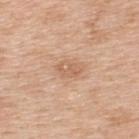This lesion was catalogued during total-body skin photography and was not selected for biopsy. From the back. A male subject aged around 50. Measured at roughly 3 mm in maximum diameter. The tile uses white-light illumination. Cropped from a total-body skin-imaging series; the visible field is about 15 mm.